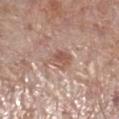Notes:
* biopsy status: total-body-photography surveillance lesion; no biopsy
* TBP lesion metrics: an outline eccentricity of about 0.7 (0 = round, 1 = elongated) and a symmetry-axis asymmetry near 0.4
* anatomic site: the leg
* imaging modality: ~15 mm crop, total-body skin-cancer survey
* lighting: white-light
* subject: male, in their 70s